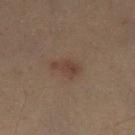Recorded during total-body skin imaging; not selected for excision or biopsy.
Captured under cross-polarized illumination.
A 15 mm close-up tile from a total-body photography series done for melanoma screening.
On the left lower leg.
The lesion-visualizer software estimated an average lesion color of about L≈31 a*≈14 b*≈19 (CIELAB), about 6 CIELAB-L* units darker than the surrounding skin, and a normalized border contrast of about 6. It also reported a border-irregularity index near 3.5/10 and peripheral color asymmetry of about 0.5. It also reported a classifier nevus-likeness of about 40/100.
The recorded lesion diameter is about 3.5 mm.
A male patient, about 55 years old.